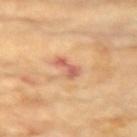  biopsy_status: not biopsied; imaged during a skin examination
  lighting: cross-polarized
  lesion_size:
    long_diameter_mm_approx: 3.0
  site: left upper arm
  patient:
    sex: female
    age_approx: 75
  image:
    source: total-body photography crop
    field_of_view_mm: 15
  automated_metrics:
    eccentricity: 0.9
    shape_asymmetry: 0.4
    cielab_L: 61
    cielab_a: 27
    cielab_b: 33
    vs_skin_darker_L: 11.0
    vs_skin_contrast_norm: 7.5
    nevus_likeness_0_100: 0
    lesion_detection_confidence_0_100: 100